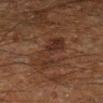Captured during whole-body skin photography for melanoma surveillance; the lesion was not biopsied.
The lesion-visualizer software estimated a lesion area of about 11 mm², an eccentricity of roughly 0.9, and two-axis asymmetry of about 0.3. The analysis additionally found a mean CIELAB color near L≈23 a*≈15 b*≈21 and a lesion–skin lightness drop of about 5. It also reported a classifier nevus-likeness of about 0/100.
A close-up tile cropped from a whole-body skin photograph, about 15 mm across.
Located on the right lower leg.
Imaged with cross-polarized lighting.
The recorded lesion diameter is about 5.5 mm.
The subject is a male aged 58–62.